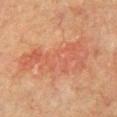Q: Was this lesion biopsied?
A: catalogued during a skin exam; not biopsied
Q: Where on the body is the lesion?
A: the left upper arm
Q: What are the patient's age and sex?
A: male, aged around 75
Q: What kind of image is this?
A: ~15 mm crop, total-body skin-cancer survey
Q: What did automated image analysis measure?
A: a lesion area of about 25 mm² and a symmetry-axis asymmetry near 0.5; a lesion color around L≈48 a*≈24 b*≈29 in CIELAB and a lesion–skin lightness drop of about 6; a border-irregularity index near 8.5/10, a color-variation rating of about 4/10, and a peripheral color-asymmetry measure near 1.5
Q: What lighting was used for the tile?
A: cross-polarized illumination